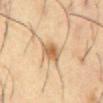notes: catalogued during a skin exam; not biopsied | subject: male, aged around 55 | tile lighting: cross-polarized illumination | anatomic site: the front of the torso | imaging modality: 15 mm crop, total-body photography | automated metrics: a mean CIELAB color near L≈61 a*≈18 b*≈38, about 12 CIELAB-L* units darker than the surrounding skin, and a lesion-to-skin contrast of about 8 (normalized; higher = more distinct); a nevus-likeness score of about 85/100 and a detector confidence of about 100 out of 100 that the crop contains a lesion | lesion diameter: ≈2.5 mm.